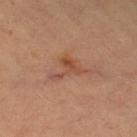| field | value |
|---|---|
| follow-up | total-body-photography surveillance lesion; no biopsy |
| patient | female, about 60 years old |
| acquisition | ~15 mm crop, total-body skin-cancer survey |
| illumination | cross-polarized |
| body site | the leg |